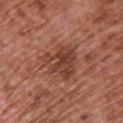notes: total-body-photography surveillance lesion; no biopsy
tile lighting: white-light
size: ≈5 mm
subject: male, aged 73 to 77
body site: the upper back
automated metrics: a within-lesion color-variation index near 5.5/10 and peripheral color asymmetry of about 2; an automated nevus-likeness rating near 25 out of 100 and a detector confidence of about 100 out of 100 that the crop contains a lesion
imaging modality: ~15 mm tile from a whole-body skin photo